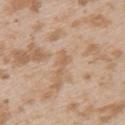Assessment:
The lesion was photographed on a routine skin check and not biopsied; there is no pathology result.
Acquisition and patient details:
The patient is a female roughly 25 years of age. The lesion is located on the left upper arm. Cropped from a total-body skin-imaging series; the visible field is about 15 mm.